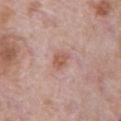Case summary:
* follow-up: no biopsy performed (imaged during a skin exam)